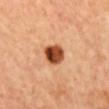Impression: The lesion was tiled from a total-body skin photograph and was not biopsied. Acquisition and patient details: About 3 mm across. A close-up tile cropped from a whole-body skin photograph, about 15 mm across. The tile uses cross-polarized illumination. A male patient aged 58–62. Located on the mid back.